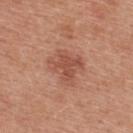The lesion was tiled from a total-body skin photograph and was not biopsied. On the upper back. A male subject aged around 30. Cropped from a whole-body photographic skin survey; the tile spans about 15 mm.